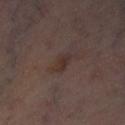| feature | finding |
|---|---|
| notes | catalogued during a skin exam; not biopsied |
| imaging modality | total-body-photography crop, ~15 mm field of view |
| patient | female, about 50 years old |
| lighting | cross-polarized illumination |
| anatomic site | the right thigh |
| lesion diameter | ≈3 mm |